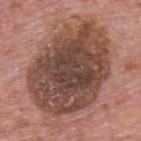Q: Was this lesion biopsied?
A: catalogued during a skin exam; not biopsied
Q: What are the patient's age and sex?
A: male, roughly 75 years of age
Q: What kind of image is this?
A: total-body-photography crop, ~15 mm field of view
Q: Where on the body is the lesion?
A: the upper back
Q: Illumination type?
A: white-light
Q: How large is the lesion?
A: about 11 mm